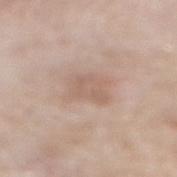Imaged during a routine full-body skin examination; the lesion was not biopsied and no histopathology is available.
The lesion's longest dimension is about 4 mm.
From the back.
A male patient, aged around 80.
The tile uses white-light illumination.
A 15 mm crop from a total-body photograph taken for skin-cancer surveillance.